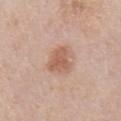The lesion was tiled from a total-body skin photograph and was not biopsied.
A roughly 15 mm field-of-view crop from a total-body skin photograph.
Longest diameter approximately 3.5 mm.
A male patient aged 58–62.
Captured under white-light illumination.
An algorithmic analysis of the crop reported an area of roughly 8 mm², a shape eccentricity near 0.5, and a shape-asymmetry score of about 0.35 (0 = symmetric). The analysis additionally found a lesion color around L≈59 a*≈21 b*≈30 in CIELAB, about 10 CIELAB-L* units darker than the surrounding skin, and a lesion-to-skin contrast of about 7 (normalized; higher = more distinct). The analysis additionally found border irregularity of about 3.5 on a 0–10 scale and a color-variation rating of about 2.5/10. The software also gave a detector confidence of about 100 out of 100 that the crop contains a lesion.
Located on the front of the torso.